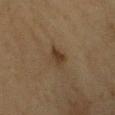No biopsy was performed on this lesion — it was imaged during a full skin examination and was not determined to be concerning. The tile uses cross-polarized illumination. This image is a 15 mm lesion crop taken from a total-body photograph. Located on the left forearm. The subject is a female roughly 55 years of age. Measured at roughly 2.5 mm in maximum diameter.